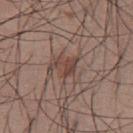notes: total-body-photography surveillance lesion; no biopsy
acquisition: ~15 mm tile from a whole-body skin photo
illumination: white-light illumination
subject: male, in their mid-30s
body site: the chest
automated lesion analysis: a mean CIELAB color near L≈43 a*≈18 b*≈23, a lesion–skin lightness drop of about 8, and a normalized border contrast of about 7; an automated nevus-likeness rating near 70 out of 100 and a lesion-detection confidence of about 95/100
lesion size: ≈3 mm